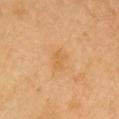Recorded during total-body skin imaging; not selected for excision or biopsy. The lesion is on the head or neck. A roughly 15 mm field-of-view crop from a total-body skin photograph. Captured under cross-polarized illumination. A female patient about 40 years old. An algorithmic analysis of the crop reported a lesion area of about 4 mm², an outline eccentricity of about 0.75 (0 = round, 1 = elongated), and two-axis asymmetry of about 0.35. It also reported roughly 6 lightness units darker than nearby skin and a normalized lesion–skin contrast near 5. The recorded lesion diameter is about 2.5 mm.